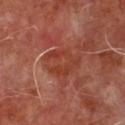workup: imaged on a skin check; not biopsied | imaging modality: total-body-photography crop, ~15 mm field of view | anatomic site: the front of the torso | automated lesion analysis: an outline eccentricity of about 0.65 (0 = round, 1 = elongated) and a symmetry-axis asymmetry near 0.35; border irregularity of about 7.5 on a 0–10 scale, a color-variation rating of about 3/10, and radial color variation of about 1 | subject: male, aged 63 to 67 | illumination: cross-polarized.